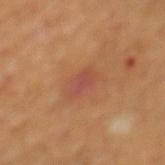Assessment: Imaged during a routine full-body skin examination; the lesion was not biopsied and no histopathology is available. Background: Captured under cross-polarized illumination. The subject is a male aged 68–72. A 15 mm close-up extracted from a 3D total-body photography capture. About 3 mm across. The lesion-visualizer software estimated two-axis asymmetry of about 0.25. It also reported a within-lesion color-variation index near 2/10 and a peripheral color-asymmetry measure near 0.5. The analysis additionally found a detector confidence of about 100 out of 100 that the crop contains a lesion. From the mid back.